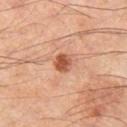Imaged during a routine full-body skin examination; the lesion was not biopsied and no histopathology is available. The lesion-visualizer software estimated a classifier nevus-likeness of about 95/100 and lesion-presence confidence of about 100/100. A male patient, aged approximately 65. This image is a 15 mm lesion crop taken from a total-body photograph. The lesion is located on the left thigh.